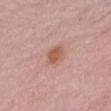The lesion was photographed on a routine skin check and not biopsied; there is no pathology result. Longest diameter approximately 2.5 mm. This is a white-light tile. This image is a 15 mm lesion crop taken from a total-body photograph. The subject is a male aged 48 to 52. Automated image analysis of the tile measured an average lesion color of about L≈57 a*≈23 b*≈28 (CIELAB), a lesion–skin lightness drop of about 9, and a normalized border contrast of about 7. The software also gave a border-irregularity rating of about 1.5/10, a color-variation rating of about 2/10, and peripheral color asymmetry of about 1. Located on the chest.